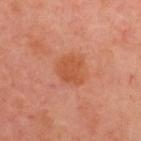Image and clinical context:
The lesion-visualizer software estimated a lesion area of about 8 mm², an eccentricity of roughly 0.5, and a symmetry-axis asymmetry near 0.2. It also reported a lesion color around L≈52 a*≈29 b*≈37 in CIELAB, about 7 CIELAB-L* units darker than the surrounding skin, and a normalized lesion–skin contrast near 6.5. The software also gave a classifier nevus-likeness of about 25/100 and a lesion-detection confidence of about 100/100. The lesion is located on the upper back. Imaged with cross-polarized lighting. Cropped from a whole-body photographic skin survey; the tile spans about 15 mm. A male subject, about 50 years old.